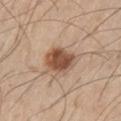{
  "automated_metrics": {
    "area_mm2_approx": 11.0,
    "eccentricity": 0.65,
    "shape_asymmetry": 0.2,
    "border_irregularity_0_10": 2.0,
    "color_variation_0_10": 5.0,
    "peripheral_color_asymmetry": 1.5,
    "nevus_likeness_0_100": 95,
    "lesion_detection_confidence_0_100": 100
  },
  "image": {
    "source": "total-body photography crop",
    "field_of_view_mm": 15
  },
  "patient": {
    "sex": "male",
    "age_approx": 60
  },
  "lesion_size": {
    "long_diameter_mm_approx": 4.5
  },
  "lighting": "white-light",
  "site": "left thigh"
}